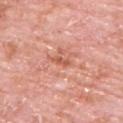The lesion was tiled from a total-body skin photograph and was not biopsied. The subject is a male approximately 80 years of age. The lesion is located on the upper back. A 15 mm close-up extracted from a 3D total-body photography capture. Longest diameter approximately 2.5 mm. Imaged with white-light lighting.